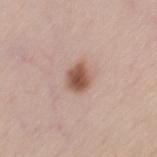  biopsy_status: not biopsied; imaged during a skin examination
  lesion_size:
    long_diameter_mm_approx: 3.0
  patient:
    sex: female
    age_approx: 55
  site: chest
  lighting: white-light
  image:
    source: total-body photography crop
    field_of_view_mm: 15
  automated_metrics:
    area_mm2_approx: 6.0
    eccentricity: 0.6
    shape_asymmetry: 0.25
    cielab_L: 54
    cielab_a: 20
    cielab_b: 27
    vs_skin_contrast_norm: 10.0
    nevus_likeness_0_100: 100